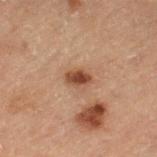Q: Was this lesion biopsied?
A: catalogued during a skin exam; not biopsied
Q: What is the imaging modality?
A: ~15 mm tile from a whole-body skin photo
Q: What did automated image analysis measure?
A: a classifier nevus-likeness of about 95/100 and lesion-presence confidence of about 100/100
Q: What is the anatomic site?
A: the leg
Q: Lesion size?
A: ≈2.5 mm
Q: Illumination type?
A: cross-polarized
Q: Patient demographics?
A: male, aged 68 to 72